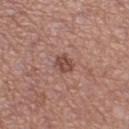Part of a total-body skin-imaging series; this lesion was reviewed on a skin check and was not flagged for biopsy. Automated tile analysis of the lesion measured a footprint of about 3.5 mm², a shape eccentricity near 0.75, and a symmetry-axis asymmetry near 0.25. The software also gave a lesion color around L≈46 a*≈23 b*≈25 in CIELAB, roughly 11 lightness units darker than nearby skin, and a normalized border contrast of about 8. And it measured a detector confidence of about 100 out of 100 that the crop contains a lesion. A 15 mm close-up tile from a total-body photography series done for melanoma screening. Approximately 2.5 mm at its widest. The lesion is on the leg. The subject is a female aged 38–42. Captured under white-light illumination.